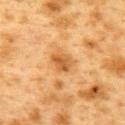Q: Was this lesion biopsied?
A: total-body-photography surveillance lesion; no biopsy
Q: How was this image acquired?
A: ~15 mm crop, total-body skin-cancer survey
Q: What are the patient's age and sex?
A: female, in their 40s
Q: What did automated image analysis measure?
A: an outline eccentricity of about 0.65 (0 = round, 1 = elongated) and a symmetry-axis asymmetry near 0.2; a lesion color around L≈49 a*≈21 b*≈39 in CIELAB, about 9 CIELAB-L* units darker than the surrounding skin, and a normalized border contrast of about 7
Q: Lesion location?
A: the upper back